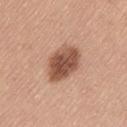patient: male, approximately 60 years of age
location: the left thigh
tile lighting: white-light
acquisition: total-body-photography crop, ~15 mm field of view
image-analysis metrics: a lesion area of about 14 mm², an eccentricity of roughly 0.8, and a shape-asymmetry score of about 0.15 (0 = symmetric); a color-variation rating of about 5/10 and a peripheral color-asymmetry measure near 1.5